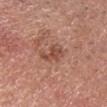About 3.5 mm across. This is a white-light tile. Cropped from a whole-body photographic skin survey; the tile spans about 15 mm. From the head or neck. The patient is a male aged around 60.